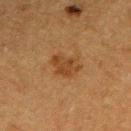The tile uses cross-polarized illumination. The lesion is located on the right upper arm. Automated tile analysis of the lesion measured an area of roughly 7.5 mm², a shape eccentricity near 0.65, and a shape-asymmetry score of about 0.25 (0 = symmetric). The analysis additionally found a classifier nevus-likeness of about 50/100 and lesion-presence confidence of about 100/100. Cropped from a total-body skin-imaging series; the visible field is about 15 mm. The subject is a male aged 73–77.